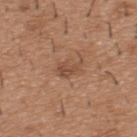Part of a total-body skin-imaging series; this lesion was reviewed on a skin check and was not flagged for biopsy. Located on the upper back. A 15 mm close-up tile from a total-body photography series done for melanoma screening. Captured under white-light illumination. Measured at roughly 3 mm in maximum diameter. The subject is a male aged approximately 40.